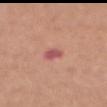Q: Was a biopsy performed?
A: imaged on a skin check; not biopsied
Q: What kind of image is this?
A: ~15 mm tile from a whole-body skin photo
Q: How large is the lesion?
A: ≈3 mm
Q: Lesion location?
A: the abdomen
Q: Illumination type?
A: white-light illumination
Q: What are the patient's age and sex?
A: male, aged 63 to 67